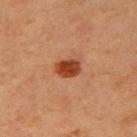Clinical impression:
Captured during whole-body skin photography for melanoma surveillance; the lesion was not biopsied.
Background:
Located on the right upper arm. The subject is a female approximately 40 years of age. Approximately 3 mm at its widest. The tile uses cross-polarized illumination. Cropped from a total-body skin-imaging series; the visible field is about 15 mm.